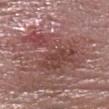Notes:
– workup — no biopsy performed (imaged during a skin exam)
– anatomic site — the head or neck
– diameter — ≈9 mm
– image source — 15 mm crop, total-body photography
– tile lighting — white-light illumination
– patient — male, about 85 years old or older